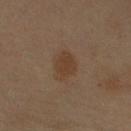{"biopsy_status": "not biopsied; imaged during a skin examination", "lighting": "cross-polarized", "image": {"source": "total-body photography crop", "field_of_view_mm": 15}, "site": "right upper arm", "automated_metrics": {"border_irregularity_0_10": 2.0, "color_variation_0_10": 2.0}, "patient": {"sex": "female", "age_approx": 60}, "lesion_size": {"long_diameter_mm_approx": 3.5}}